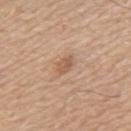Impression:
Recorded during total-body skin imaging; not selected for excision or biopsy.
Image and clinical context:
Longest diameter approximately 2.5 mm. The patient is a male aged approximately 65. The tile uses white-light illumination. The lesion is on the upper back. A 15 mm crop from a total-body photograph taken for skin-cancer surveillance.